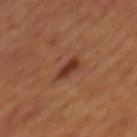Notes:
- follow-up — catalogued during a skin exam; not biopsied
- lesion diameter — ~3 mm (longest diameter)
- acquisition — 15 mm crop, total-body photography
- site — the back
- lighting — cross-polarized
- subject — male, roughly 65 years of age
- TBP lesion metrics — a lesion area of about 3.5 mm² and a shape-asymmetry score of about 0.2 (0 = symmetric); peripheral color asymmetry of about 1; a lesion-detection confidence of about 100/100